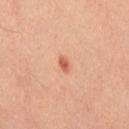No biopsy was performed on this lesion — it was imaged during a full skin examination and was not determined to be concerning. The subject is a male in their mid- to late 40s. A roughly 15 mm field-of-view crop from a total-body skin photograph. The lesion is located on the back. The total-body-photography lesion software estimated an area of roughly 2 mm² and an eccentricity of roughly 0.8. The software also gave roughly 11 lightness units darker than nearby skin and a normalized border contrast of about 8. The software also gave an automated nevus-likeness rating near 90 out of 100 and a detector confidence of about 100 out of 100 that the crop contains a lesion. Imaged with cross-polarized lighting.